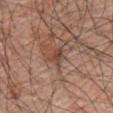follow-up = no biopsy performed (imaged during a skin exam) | body site = the front of the torso | image source = ~15 mm crop, total-body skin-cancer survey | patient = male, in their 70s.